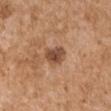{
  "biopsy_status": "not biopsied; imaged during a skin examination",
  "automated_metrics": {
    "color_variation_0_10": 3.5,
    "peripheral_color_asymmetry": 1.5,
    "nevus_likeness_0_100": 45,
    "lesion_detection_confidence_0_100": 100
  },
  "patient": {
    "sex": "male",
    "age_approx": 75
  },
  "lesion_size": {
    "long_diameter_mm_approx": 3.0
  },
  "site": "arm",
  "image": {
    "source": "total-body photography crop",
    "field_of_view_mm": 15
  },
  "lighting": "white-light"
}